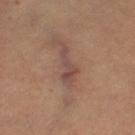Clinical impression: The lesion was tiled from a total-body skin photograph and was not biopsied. Context: The tile uses cross-polarized illumination. The recorded lesion diameter is about 4.5 mm. The lesion is on the left thigh. This image is a 15 mm lesion crop taken from a total-body photograph. A female patient, roughly 70 years of age.